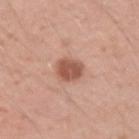The lesion was tiled from a total-body skin photograph and was not biopsied. On the left upper arm. A male patient approximately 30 years of age. This is a white-light tile. A 15 mm close-up tile from a total-body photography series done for melanoma screening.